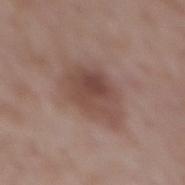This image is a 15 mm lesion crop taken from a total-body photograph. A female subject, aged around 70. The tile uses white-light illumination. The total-body-photography lesion software estimated a lesion-detection confidence of about 100/100. Located on the mid back. The recorded lesion diameter is about 5.5 mm.